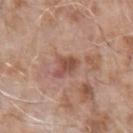  site: left upper arm
  patient:
    sex: male
    age_approx: 75
  automated_metrics:
    area_mm2_approx: 5.0
    shape_asymmetry: 0.35
    cielab_L: 50
    cielab_a: 23
    cielab_b: 27
    vs_skin_darker_L: 11.0
    vs_skin_contrast_norm: 7.5
    border_irregularity_0_10: 3.5
    color_variation_0_10: 2.0
    peripheral_color_asymmetry: 0.5
    nevus_likeness_0_100: 0
  image:
    source: total-body photography crop
    field_of_view_mm: 15
  lighting: white-light
  lesion_size:
    long_diameter_mm_approx: 3.0